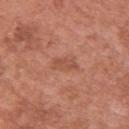This lesion was catalogued during total-body skin photography and was not selected for biopsy. This image is a 15 mm lesion crop taken from a total-body photograph. Measured at roughly 3 mm in maximum diameter. The tile uses white-light illumination. On the upper back. A female subject, aged around 35.